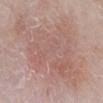Captured during whole-body skin photography for melanoma surveillance; the lesion was not biopsied. Located on the right lower leg. A male patient, aged 63 to 67. The lesion's longest dimension is about 11.5 mm. The tile uses white-light illumination. An algorithmic analysis of the crop reported a within-lesion color-variation index near 4/10 and radial color variation of about 1. A 15 mm crop from a total-body photograph taken for skin-cancer surveillance.